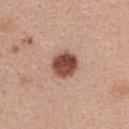biopsy status = imaged on a skin check; not biopsied
lesion diameter = ≈3.5 mm
subject = female, roughly 40 years of age
tile lighting = white-light illumination
imaging modality = ~15 mm crop, total-body skin-cancer survey
site = the upper back